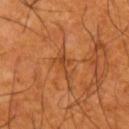Impression:
The lesion was photographed on a routine skin check and not biopsied; there is no pathology result.
Acquisition and patient details:
A male patient aged 63–67. The total-body-photography lesion software estimated an area of roughly 3.5 mm², an outline eccentricity of about 0.9 (0 = round, 1 = elongated), and two-axis asymmetry of about 0.55. The analysis additionally found border irregularity of about 7 on a 0–10 scale and a peripheral color-asymmetry measure near 0. And it measured a classifier nevus-likeness of about 0/100. The tile uses cross-polarized illumination. The lesion is located on the arm. The lesion's longest dimension is about 3 mm. A 15 mm close-up tile from a total-body photography series done for melanoma screening.